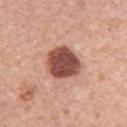Clinical impression: This lesion was catalogued during total-body skin photography and was not selected for biopsy. Context: Approximately 4.5 mm at its widest. A close-up tile cropped from a whole-body skin photograph, about 15 mm across. Located on the arm. Imaged with white-light lighting. Automated image analysis of the tile measured a lesion area of about 13 mm², an outline eccentricity of about 0.45 (0 = round, 1 = elongated), and two-axis asymmetry of about 0.2. It also reported a border-irregularity rating of about 1.5/10, a color-variation rating of about 4/10, and peripheral color asymmetry of about 1. And it measured an automated nevus-likeness rating near 70 out of 100 and a lesion-detection confidence of about 100/100. A female subject aged around 60.